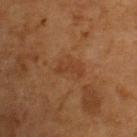Clinical impression: Captured during whole-body skin photography for melanoma surveillance; the lesion was not biopsied. Image and clinical context: The patient is a female in their 40s. The lesion's longest dimension is about 3.5 mm. Cropped from a whole-body photographic skin survey; the tile spans about 15 mm. On the upper back.